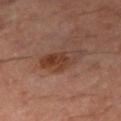<case>
  <biopsy_status>not biopsied; imaged during a skin examination</biopsy_status>
  <lesion_size>
    <long_diameter_mm_approx>5.5</long_diameter_mm_approx>
  </lesion_size>
  <site>right thigh</site>
  <lighting>cross-polarized</lighting>
  <image>
    <source>total-body photography crop</source>
    <field_of_view_mm>15</field_of_view_mm>
  </image>
  <automated_metrics>
    <eccentricity>0.9</eccentricity>
    <nevus_likeness_0_100>30</nevus_likeness_0_100>
    <lesion_detection_confidence_0_100>100</lesion_detection_confidence_0_100>
  </automated_metrics>
  <patient>
    <sex>male</sex>
    <age_approx>60</age_approx>
  </patient>
</case>